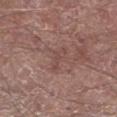follow-up — total-body-photography surveillance lesion; no biopsy
lesion size — ~3.5 mm (longest diameter)
anatomic site — the left lower leg
TBP lesion metrics — internal color variation of about 0 on a 0–10 scale; a nevus-likeness score of about 0/100
lighting — white-light illumination
subject — male, roughly 70 years of age
image — 15 mm crop, total-body photography